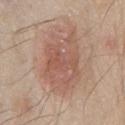workup: total-body-photography surveillance lesion; no biopsy | subject: male, about 80 years old | imaging modality: ~15 mm crop, total-body skin-cancer survey | lesion size: about 8 mm | anatomic site: the chest | illumination: white-light illumination.